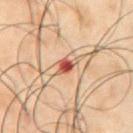Q: Was this lesion biopsied?
A: total-body-photography surveillance lesion; no biopsy
Q: Illumination type?
A: cross-polarized illumination
Q: What is the anatomic site?
A: the abdomen
Q: What are the patient's age and sex?
A: male, in their 50s
Q: What kind of image is this?
A: ~15 mm crop, total-body skin-cancer survey